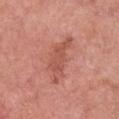follow-up — no biopsy performed (imaged during a skin exam)
lesion diameter — ≈6 mm
patient — female, roughly 70 years of age
lighting — white-light illumination
anatomic site — the chest
imaging modality — total-body-photography crop, ~15 mm field of view